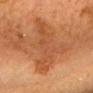{"biopsy_status": "not biopsied; imaged during a skin examination", "automated_metrics": {"vs_skin_contrast_norm": 5.5}, "lighting": "cross-polarized", "image": {"source": "total-body photography crop", "field_of_view_mm": 15}, "patient": {"sex": "male", "age_approx": "85+"}, "site": "head or neck", "lesion_size": {"long_diameter_mm_approx": 10.0}}